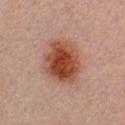Captured during whole-body skin photography for melanoma surveillance; the lesion was not biopsied. From the chest. About 5.5 mm across. A 15 mm close-up extracted from a 3D total-body photography capture. The subject is a male roughly 30 years of age. Imaged with cross-polarized lighting.